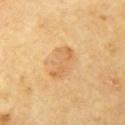The lesion's longest dimension is about 4 mm. A roughly 15 mm field-of-view crop from a total-body skin photograph. This is a cross-polarized tile. Located on the left upper arm. A male subject aged approximately 70.